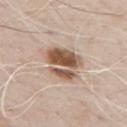Impression:
Imaged during a routine full-body skin examination; the lesion was not biopsied and no histopathology is available.
Context:
A male patient, in their 70s. Imaged with white-light lighting. A 15 mm close-up tile from a total-body photography series done for melanoma screening. The lesion is on the chest.